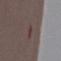Impression:
Part of a total-body skin-imaging series; this lesion was reviewed on a skin check and was not flagged for biopsy.
Acquisition and patient details:
A female patient, about 30 years old. A roughly 15 mm field-of-view crop from a total-body skin photograph. The lesion is located on the arm. Imaged with white-light lighting. The lesion's longest dimension is about 3 mm. The total-body-photography lesion software estimated a lesion area of about 1.5 mm², an outline eccentricity of about 0.95 (0 = round, 1 = elongated), and a symmetry-axis asymmetry near 0.4. And it measured a lesion color around L≈37 a*≈17 b*≈16 in CIELAB, about 5 CIELAB-L* units darker than the surrounding skin, and a normalized lesion–skin contrast near 5. And it measured a border-irregularity index near 5.5/10 and peripheral color asymmetry of about 0.